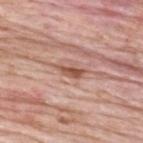Clinical impression:
The lesion was tiled from a total-body skin photograph and was not biopsied.
Context:
A male patient aged 58 to 62. Measured at roughly 3 mm in maximum diameter. A 15 mm close-up tile from a total-body photography series done for melanoma screening. From the back.